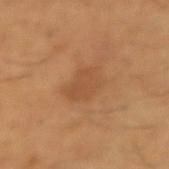Assessment:
Recorded during total-body skin imaging; not selected for excision or biopsy.
Background:
A roughly 15 mm field-of-view crop from a total-body skin photograph. A male subject, in their mid-60s. The lesion-visualizer software estimated an average lesion color of about L≈50 a*≈22 b*≈37 (CIELAB), about 6 CIELAB-L* units darker than the surrounding skin, and a normalized border contrast of about 4.5. It also reported a border-irregularity rating of about 2.5/10 and peripheral color asymmetry of about 0.5. And it measured an automated nevus-likeness rating near 0 out of 100 and a lesion-detection confidence of about 100/100. Approximately 4 mm at its widest. From the right forearm. The tile uses cross-polarized illumination.